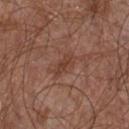No biopsy was performed on this lesion — it was imaged during a full skin examination and was not determined to be concerning. The lesion's longest dimension is about 3.5 mm. The lesion is located on the chest. Imaged with white-light lighting. The patient is a male aged approximately 55. A close-up tile cropped from a whole-body skin photograph, about 15 mm across.